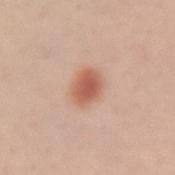This lesion was catalogued during total-body skin photography and was not selected for biopsy.
About 3.5 mm across.
Captured under white-light illumination.
A 15 mm crop from a total-body photograph taken for skin-cancer surveillance.
Located on the arm.
The subject is a female about 25 years old.